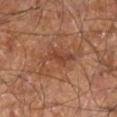A 15 mm crop from a total-body photograph taken for skin-cancer surveillance.
The total-body-photography lesion software estimated a mean CIELAB color near L≈43 a*≈22 b*≈30, about 7 CIELAB-L* units darker than the surrounding skin, and a normalized lesion–skin contrast near 6. And it measured a border-irregularity index near 9/10 and peripheral color asymmetry of about 1.
Longest diameter approximately 6 mm.
The subject is a male aged 58 to 62.
On the leg.